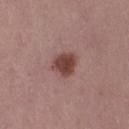{
  "biopsy_status": "not biopsied; imaged during a skin examination",
  "patient": {
    "sex": "female",
    "age_approx": 50
  },
  "site": "right thigh",
  "image": {
    "source": "total-body photography crop",
    "field_of_view_mm": 15
  }
}